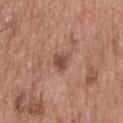• follow-up: imaged on a skin check; not biopsied
• illumination: white-light
• lesion diameter: ≈3 mm
• body site: the mid back
• imaging modality: ~15 mm tile from a whole-body skin photo
• patient: male, aged 48–52
• automated metrics: a color-variation rating of about 3/10 and peripheral color asymmetry of about 1; lesion-presence confidence of about 100/100